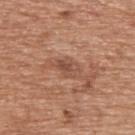<record>
  <biopsy_status>not biopsied; imaged during a skin examination</biopsy_status>
  <lesion_size>
    <long_diameter_mm_approx>3.5</long_diameter_mm_approx>
  </lesion_size>
  <automated_metrics>
    <area_mm2_approx>5.5</area_mm2_approx>
    <shape_asymmetry>0.3</shape_asymmetry>
  </automated_metrics>
  <lighting>white-light</lighting>
  <site>back</site>
  <image>
    <source>total-body photography crop</source>
    <field_of_view_mm>15</field_of_view_mm>
  </image>
  <patient>
    <sex>male</sex>
    <age_approx>75</age_approx>
  </patient>
</record>